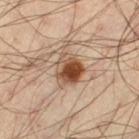Captured during whole-body skin photography for melanoma surveillance; the lesion was not biopsied. A 15 mm close-up extracted from a 3D total-body photography capture. A male subject aged 33 to 37. About 3.5 mm across. This is a cross-polarized tile. On the left thigh. Automated image analysis of the tile measured an eccentricity of roughly 0.5 and a symmetry-axis asymmetry near 0.2.